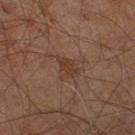Notes:
- workup · catalogued during a skin exam; not biopsied
- imaging modality · ~15 mm crop, total-body skin-cancer survey
- automated metrics · an automated nevus-likeness rating near 0 out of 100
- location · the right leg
- lighting · cross-polarized
- subject · male, aged approximately 60
- lesion size · about 2.5 mm